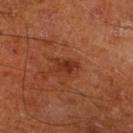Q: Was this lesion biopsied?
A: imaged on a skin check; not biopsied
Q: Who is the patient?
A: male, approximately 65 years of age
Q: How large is the lesion?
A: ~3 mm (longest diameter)
Q: Where on the body is the lesion?
A: the right lower leg
Q: How was this image acquired?
A: total-body-photography crop, ~15 mm field of view
Q: How was the tile lit?
A: cross-polarized
Q: What did automated image analysis measure?
A: a lesion area of about 3.5 mm², a shape eccentricity near 0.8, and a symmetry-axis asymmetry near 0.45; an average lesion color of about L≈31 a*≈27 b*≈32 (CIELAB) and roughly 8 lightness units darker than nearby skin; a classifier nevus-likeness of about 25/100 and a detector confidence of about 100 out of 100 that the crop contains a lesion